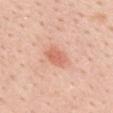Q: Was this lesion biopsied?
A: no biopsy performed (imaged during a skin exam)
Q: Lesion size?
A: ~3.5 mm (longest diameter)
Q: What are the patient's age and sex?
A: female, aged 43–47
Q: Illumination type?
A: white-light illumination
Q: What kind of image is this?
A: 15 mm crop, total-body photography
Q: What is the anatomic site?
A: the upper back